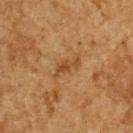follow-up: total-body-photography surveillance lesion; no biopsy
image-analysis metrics: a lesion area of about 4 mm², a shape eccentricity near 0.9, and a symmetry-axis asymmetry near 0.4
location: the upper back
diameter: ≈3.5 mm
image source: ~15 mm tile from a whole-body skin photo
illumination: cross-polarized
subject: male, in their mid- to late 80s or older